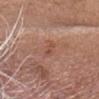This lesion was catalogued during total-body skin photography and was not selected for biopsy.
A male patient in their mid-70s.
The tile uses white-light illumination.
Measured at roughly 3 mm in maximum diameter.
The lesion is located on the head or neck.
This image is a 15 mm lesion crop taken from a total-body photograph.
An algorithmic analysis of the crop reported a border-irregularity index near 3.5/10, internal color variation of about 1 on a 0–10 scale, and radial color variation of about 0. The software also gave an automated nevus-likeness rating near 0 out of 100.